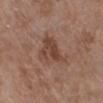Clinical impression:
Part of a total-body skin-imaging series; this lesion was reviewed on a skin check and was not flagged for biopsy.
Acquisition and patient details:
A female patient, approximately 65 years of age. The lesion is on the right lower leg. The tile uses white-light illumination. The recorded lesion diameter is about 4.5 mm. A 15 mm close-up extracted from a 3D total-body photography capture.